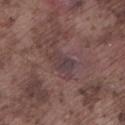Findings:
- workup — no biopsy performed (imaged during a skin exam)